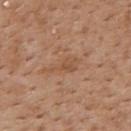| field | value |
|---|---|
| follow-up | total-body-photography surveillance lesion; no biopsy |
| site | the upper back |
| lighting | white-light illumination |
| automated lesion analysis | a peripheral color-asymmetry measure near 0.5 |
| acquisition | total-body-photography crop, ~15 mm field of view |
| lesion size | ~4 mm (longest diameter) |
| patient | male, about 60 years old |